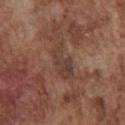Part of a total-body skin-imaging series; this lesion was reviewed on a skin check and was not flagged for biopsy.
A male patient, approximately 75 years of age.
Approximately 4.5 mm at its widest.
The lesion is located on the chest.
The tile uses white-light illumination.
Cropped from a total-body skin-imaging series; the visible field is about 15 mm.